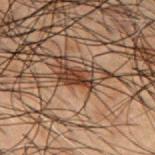Impression: Imaged during a routine full-body skin examination; the lesion was not biopsied and no histopathology is available. Background: The patient is a male aged 48–52. Automated tile analysis of the lesion measured a lesion area of about 5 mm², an outline eccentricity of about 0.9 (0 = round, 1 = elongated), and two-axis asymmetry of about 0.2. The recorded lesion diameter is about 3.5 mm. From the upper back. Cropped from a whole-body photographic skin survey; the tile spans about 15 mm.